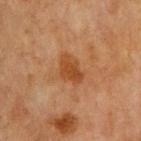The lesion was tiled from a total-body skin photograph and was not biopsied.
A male subject about 65 years old.
Cropped from a total-body skin-imaging series; the visible field is about 15 mm.
The lesion is located on the front of the torso.